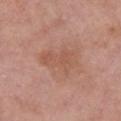Notes:
• follow-up · total-body-photography surveillance lesion; no biopsy
• tile lighting · white-light illumination
• subject · female, aged 58 to 62
• location · the chest
• imaging modality · total-body-photography crop, ~15 mm field of view
• lesion diameter · about 5 mm